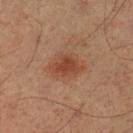No biopsy was performed on this lesion — it was imaged during a full skin examination and was not determined to be concerning. The subject is a male in their mid-50s. About 4.5 mm across. This image is a 15 mm lesion crop taken from a total-body photograph. The lesion is located on the left lower leg.